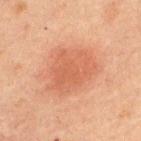Background: From the back. Imaged with cross-polarized lighting. The patient is a male about 60 years old. Longest diameter approximately 6.5 mm. Cropped from a whole-body photographic skin survey; the tile spans about 15 mm.